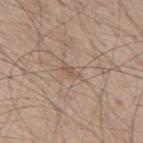tile lighting: white-light illumination
site: the upper back
size: ~3 mm (longest diameter)
patient: male, aged approximately 65
acquisition: ~15 mm crop, total-body skin-cancer survey